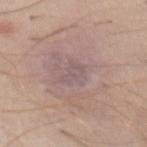workup — no biopsy performed (imaged during a skin exam) | patient — male, aged 38 to 42 | location — the abdomen | image source — ~15 mm crop, total-body skin-cancer survey.